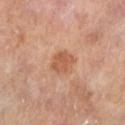Clinical summary: Located on the left lower leg. This image is a 15 mm lesion crop taken from a total-body photograph. A male subject roughly 65 years of age. Approximately 3.5 mm at its widest. This is a cross-polarized tile.